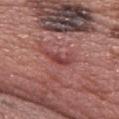notes = no biopsy performed (imaged during a skin exam) | patient = female, aged 58–62 | size = ~3.5 mm (longest diameter) | anatomic site = the upper back | image source = 15 mm crop, total-body photography.